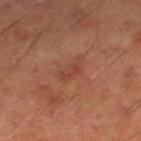The lesion is located on the right lower leg. This is a cross-polarized tile. A male patient, aged 58 to 62. A 15 mm close-up extracted from a 3D total-body photography capture.